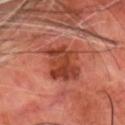{"biopsy_status": "not biopsied; imaged during a skin examination", "site": "head or neck", "image": {"source": "total-body photography crop", "field_of_view_mm": 15}, "patient": {"sex": "male", "age_approx": 70}, "automated_metrics": {"cielab_L": 42, "cielab_a": 30, "cielab_b": 32, "border_irregularity_0_10": 3.5, "color_variation_0_10": 5.5, "lesion_detection_confidence_0_100": 100}, "lighting": "cross-polarized", "lesion_size": {"long_diameter_mm_approx": 6.5}}